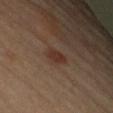Captured during whole-body skin photography for melanoma surveillance; the lesion was not biopsied. This is a cross-polarized tile. The lesion's longest dimension is about 3 mm. Located on the right upper arm. A female patient aged approximately 60. A 15 mm close-up extracted from a 3D total-body photography capture.